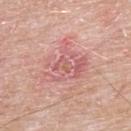The lesion was tiled from a total-body skin photograph and was not biopsied.
The tile uses white-light illumination.
Cropped from a total-body skin-imaging series; the visible field is about 15 mm.
Approximately 5.5 mm at its widest.
On the back.
The patient is a male about 65 years old.